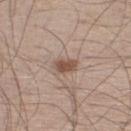Q: Was this lesion biopsied?
A: no biopsy performed (imaged during a skin exam)
Q: What lighting was used for the tile?
A: white-light
Q: Lesion size?
A: ~2.5 mm (longest diameter)
Q: What is the imaging modality?
A: ~15 mm tile from a whole-body skin photo
Q: Where on the body is the lesion?
A: the right lower leg
Q: What are the patient's age and sex?
A: male, aged 58 to 62